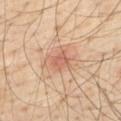Image and clinical context:
Longest diameter approximately 2.5 mm. A male patient, aged 38 to 42. On the upper back. Automated image analysis of the tile measured an area of roughly 3.5 mm² and a shape eccentricity near 0.65. The analysis additionally found a mean CIELAB color near L≈60 a*≈25 b*≈31, roughly 9 lightness units darker than nearby skin, and a normalized border contrast of about 5.5. It also reported an automated nevus-likeness rating near 75 out of 100. A region of skin cropped from a whole-body photographic capture, roughly 15 mm wide. Captured under cross-polarized illumination.